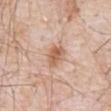Part of a total-body skin-imaging series; this lesion was reviewed on a skin check and was not flagged for biopsy.
Captured under white-light illumination.
A male subject aged around 80.
About 3 mm across.
This image is a 15 mm lesion crop taken from a total-body photograph.
On the abdomen.
The lesion-visualizer software estimated a lesion area of about 6.5 mm², a shape eccentricity near 0.5, and two-axis asymmetry of about 0.2. The software also gave a border-irregularity index near 2/10 and peripheral color asymmetry of about 1. And it measured a nevus-likeness score of about 80/100 and a lesion-detection confidence of about 100/100.